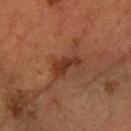biopsy status: imaged on a skin check; not biopsied | image source: 15 mm crop, total-body photography | lesion size: ~3.5 mm (longest diameter) | tile lighting: cross-polarized | subject: female, aged around 60 | body site: the left forearm.